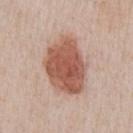notes = total-body-photography surveillance lesion; no biopsy | image source = total-body-photography crop, ~15 mm field of view | illumination = white-light | lesion diameter = about 7 mm | patient = male, aged 58–62 | site = the chest.